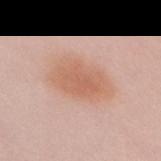Imaged during a routine full-body skin examination; the lesion was not biopsied and no histopathology is available. Automated tile analysis of the lesion measured an area of roughly 20 mm² and a symmetry-axis asymmetry near 0.15. The software also gave a border-irregularity index near 2/10, a within-lesion color-variation index near 3/10, and peripheral color asymmetry of about 1. The software also gave a nevus-likeness score of about 100/100 and lesion-presence confidence of about 100/100. Imaged with white-light lighting. A female subject aged 58 to 62. A 15 mm close-up extracted from a 3D total-body photography capture. The lesion is on the chest.